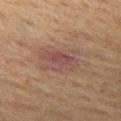This lesion was catalogued during total-body skin photography and was not selected for biopsy.
A 15 mm close-up extracted from a 3D total-body photography capture.
Measured at roughly 6.5 mm in maximum diameter.
A male patient, in their mid- to late 60s.
On the leg.